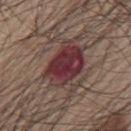Context:
This image is a 15 mm lesion crop taken from a total-body photograph. The lesion is located on the mid back. A male patient in their 70s. The tile uses white-light illumination.